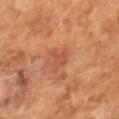follow-up = total-body-photography surveillance lesion; no biopsy | image = 15 mm crop, total-body photography | tile lighting = cross-polarized illumination | subject = male, aged approximately 65.